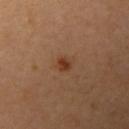Cropped from a total-body skin-imaging series; the visible field is about 15 mm. A female subject, approximately 30 years of age. Located on the left upper arm.